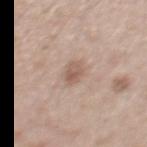The lesion was photographed on a routine skin check and not biopsied; there is no pathology result.
Cropped from a total-body skin-imaging series; the visible field is about 15 mm.
The tile uses white-light illumination.
A male subject aged 53–57.
From the abdomen.
An algorithmic analysis of the crop reported a footprint of about 4 mm² and an outline eccentricity of about 0.75 (0 = round, 1 = elongated). It also reported an average lesion color of about L≈57 a*≈17 b*≈26 (CIELAB), roughly 10 lightness units darker than nearby skin, and a lesion-to-skin contrast of about 6.5 (normalized; higher = more distinct).
The recorded lesion diameter is about 3 mm.